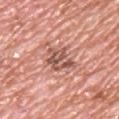Case summary:
- notes · catalogued during a skin exam; not biopsied
- patient · male, approximately 70 years of age
- site · the upper back
- lighting · white-light illumination
- diameter · about 3.5 mm
- image source · ~15 mm tile from a whole-body skin photo